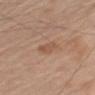<tbp_lesion>
<biopsy_status>not biopsied; imaged during a skin examination</biopsy_status>
<patient>
  <sex>male</sex>
  <age_approx>65</age_approx>
</patient>
<image>
  <source>total-body photography crop</source>
  <field_of_view_mm>15</field_of_view_mm>
</image>
<lesion_size>
  <long_diameter_mm_approx>3.5</long_diameter_mm_approx>
</lesion_size>
<site>left upper arm</site>
</tbp_lesion>